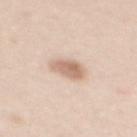Q: Is there a histopathology result?
A: imaged on a skin check; not biopsied
Q: What are the patient's age and sex?
A: female, in their 40s
Q: What is the imaging modality?
A: ~15 mm crop, total-body skin-cancer survey
Q: Illumination type?
A: white-light illumination
Q: Lesion size?
A: ≈3.5 mm
Q: What is the anatomic site?
A: the mid back